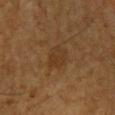A 15 mm crop from a total-body photograph taken for skin-cancer surveillance. A male patient about 60 years old. Located on the chest. About 3 mm across.